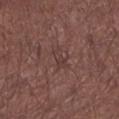Q: Was a biopsy performed?
A: no biopsy performed (imaged during a skin exam)
Q: What kind of image is this?
A: total-body-photography crop, ~15 mm field of view
Q: Where on the body is the lesion?
A: the left lower leg
Q: Who is the patient?
A: male, in their mid- to late 50s